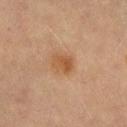biopsy_status: not biopsied; imaged during a skin examination
lesion_size:
  long_diameter_mm_approx: 2.5
patient:
  sex: male
  age_approx: 70
automated_metrics:
  color_variation_0_10: 3.5
site: left thigh
image:
  source: total-body photography crop
  field_of_view_mm: 15
lighting: cross-polarized